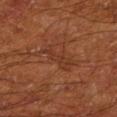| key | value |
|---|---|
| biopsy status | total-body-photography surveillance lesion; no biopsy |
| tile lighting | cross-polarized |
| subject | male, about 65 years old |
| TBP lesion metrics | an automated nevus-likeness rating near 0 out of 100 and lesion-presence confidence of about 95/100 |
| location | the leg |
| acquisition | ~15 mm crop, total-body skin-cancer survey |
| size | ~4.5 mm (longest diameter) |